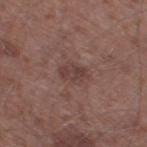Clinical impression: The lesion was photographed on a routine skin check and not biopsied; there is no pathology result. Context: This is a white-light tile. A 15 mm close-up tile from a total-body photography series done for melanoma screening. The lesion is on the left thigh. The lesion-visualizer software estimated roughly 7 lightness units darker than nearby skin and a normalized lesion–skin contrast near 6. The analysis additionally found a detector confidence of about 100 out of 100 that the crop contains a lesion. The subject is a male aged 58 to 62.